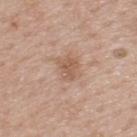Assessment: The lesion was photographed on a routine skin check and not biopsied; there is no pathology result. Image and clinical context: The lesion is on the back. An algorithmic analysis of the crop reported an eccentricity of roughly 0.65 and a symmetry-axis asymmetry near 0.3. It also reported a border-irregularity index near 3/10, a within-lesion color-variation index near 2/10, and a peripheral color-asymmetry measure near 0.5. The subject is a male approximately 60 years of age. A region of skin cropped from a whole-body photographic capture, roughly 15 mm wide.